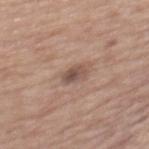Recorded during total-body skin imaging; not selected for excision or biopsy.
The lesion is on the mid back.
About 2.5 mm across.
The tile uses white-light illumination.
The lesion-visualizer software estimated a border-irregularity index near 2.5/10, internal color variation of about 4.5 on a 0–10 scale, and peripheral color asymmetry of about 2. The analysis additionally found an automated nevus-likeness rating near 0 out of 100.
A close-up tile cropped from a whole-body skin photograph, about 15 mm across.
The patient is a female aged 63–67.